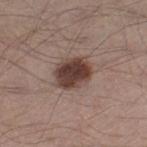The lesion was photographed on a routine skin check and not biopsied; there is no pathology result. The lesion is on the leg. The tile uses white-light illumination. Longest diameter approximately 4.5 mm. A male patient, in their 40s. A region of skin cropped from a whole-body photographic capture, roughly 15 mm wide. An algorithmic analysis of the crop reported a border-irregularity rating of about 1.5/10 and internal color variation of about 4.5 on a 0–10 scale.